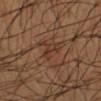Q: Was a biopsy performed?
A: catalogued during a skin exam; not biopsied
Q: Patient demographics?
A: male, about 55 years old
Q: What kind of image is this?
A: total-body-photography crop, ~15 mm field of view
Q: What lighting was used for the tile?
A: cross-polarized
Q: Where on the body is the lesion?
A: the left forearm
Q: How large is the lesion?
A: ~3.5 mm (longest diameter)